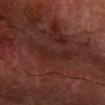Q: Is there a histopathology result?
A: imaged on a skin check; not biopsied
Q: What are the patient's age and sex?
A: male, roughly 80 years of age
Q: What is the anatomic site?
A: the arm
Q: What kind of image is this?
A: ~15 mm crop, total-body skin-cancer survey
Q: Lesion size?
A: ~6 mm (longest diameter)
Q: What did automated image analysis measure?
A: a lesion area of about 9.5 mm² and a symmetry-axis asymmetry near 0.55